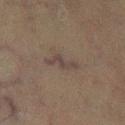– workup — no biopsy performed (imaged during a skin exam)
– size — ~4 mm (longest diameter)
– lighting — cross-polarized illumination
– image — 15 mm crop, total-body photography
– subject — male, about 65 years old
– automated lesion analysis — an area of roughly 3.5 mm² and a shape eccentricity near 0.95; a mean CIELAB color near L≈33 a*≈11 b*≈16, about 6 CIELAB-L* units darker than the surrounding skin, and a lesion-to-skin contrast of about 7 (normalized; higher = more distinct); a border-irregularity index near 7/10, internal color variation of about 0 on a 0–10 scale, and radial color variation of about 0
– body site — the left lower leg